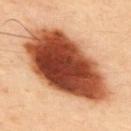Part of a total-body skin-imaging series; this lesion was reviewed on a skin check and was not flagged for biopsy. A lesion tile, about 15 mm wide, cut from a 3D total-body photograph. A male patient about 50 years old. Imaged with cross-polarized lighting. The lesion is located on the upper back. About 11.5 mm across.